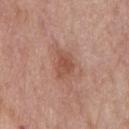Impression: Captured during whole-body skin photography for melanoma surveillance; the lesion was not biopsied. Context: A male patient, in their mid-50s. The tile uses white-light illumination. Approximately 3.5 mm at its widest. The lesion is on the chest. A lesion tile, about 15 mm wide, cut from a 3D total-body photograph.